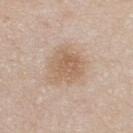Clinical impression:
This lesion was catalogued during total-body skin photography and was not selected for biopsy.
Acquisition and patient details:
An algorithmic analysis of the crop reported a lesion area of about 14 mm², a shape eccentricity near 0.6, and two-axis asymmetry of about 0.25. The software also gave a lesion–skin lightness drop of about 9 and a lesion-to-skin contrast of about 6.5 (normalized; higher = more distinct). This is a white-light tile. A male subject, roughly 35 years of age. Measured at roughly 5 mm in maximum diameter. A 15 mm close-up tile from a total-body photography series done for melanoma screening. On the upper back.